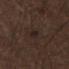Assessment: No biopsy was performed on this lesion — it was imaged during a full skin examination and was not determined to be concerning. Acquisition and patient details: The recorded lesion diameter is about 3.5 mm. Cropped from a whole-body photographic skin survey; the tile spans about 15 mm. The lesion is on the leg. The subject is a male approximately 65 years of age.